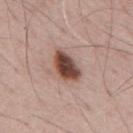Imaged during a routine full-body skin examination; the lesion was not biopsied and no histopathology is available.
Automated tile analysis of the lesion measured a lesion–skin lightness drop of about 19.
The lesion is on the back.
A male subject, aged around 70.
The tile uses white-light illumination.
A close-up tile cropped from a whole-body skin photograph, about 15 mm across.